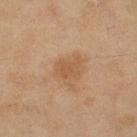Recorded during total-body skin imaging; not selected for excision or biopsy. On the right thigh. Captured under cross-polarized illumination. Automated tile analysis of the lesion measured a footprint of about 5 mm², an eccentricity of roughly 0.65, and a shape-asymmetry score of about 0.25 (0 = symmetric). The software also gave roughly 6 lightness units darker than nearby skin and a normalized border contrast of about 5.5. A 15 mm close-up tile from a total-body photography series done for melanoma screening. A female patient in their 60s. Approximately 3 mm at its widest.